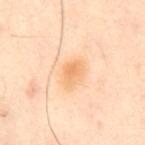| field | value |
|---|---|
| follow-up | catalogued during a skin exam; not biopsied |
| lesion diameter | ~3 mm (longest diameter) |
| illumination | cross-polarized |
| imaging modality | ~15 mm crop, total-body skin-cancer survey |
| subject | male, about 55 years old |
| automated lesion analysis | an area of roughly 5.5 mm² and an eccentricity of roughly 0.75; a mean CIELAB color near L≈76 a*≈22 b*≈43, a lesion–skin lightness drop of about 8, and a normalized lesion–skin contrast near 6.5; a border-irregularity rating of about 2/10, a color-variation rating of about 3/10, and a peripheral color-asymmetry measure near 1 |
| site | the chest |